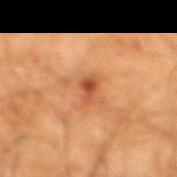workup=catalogued during a skin exam; not biopsied